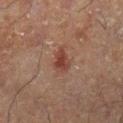{
  "biopsy_status": "not biopsied; imaged during a skin examination",
  "site": "left leg",
  "image": {
    "source": "total-body photography crop",
    "field_of_view_mm": 15
  },
  "patient": {
    "sex": "male",
    "age_approx": 60
  },
  "lighting": "cross-polarized",
  "lesion_size": {
    "long_diameter_mm_approx": 3.0
  }
}